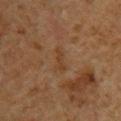Captured under cross-polarized illumination. A lesion tile, about 15 mm wide, cut from a 3D total-body photograph. A male patient, roughly 60 years of age. About 3.5 mm across. Automated image analysis of the tile measured a classifier nevus-likeness of about 0/100 and lesion-presence confidence of about 90/100. The lesion is located on the arm.